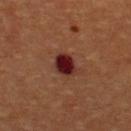Assessment:
The lesion was tiled from a total-body skin photograph and was not biopsied.